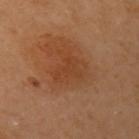Impression: The lesion was tiled from a total-body skin photograph and was not biopsied. Acquisition and patient details: A lesion tile, about 15 mm wide, cut from a 3D total-body photograph. The lesion is located on the arm. About 4 mm across. A female subject, aged approximately 60. Captured under cross-polarized illumination.